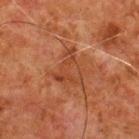Imaged during a routine full-body skin examination; the lesion was not biopsied and no histopathology is available. Approximately 4 mm at its widest. A male subject roughly 80 years of age. From the upper back. Automated tile analysis of the lesion measured a normalized border contrast of about 5.5. The software also gave a border-irregularity index near 7/10 and peripheral color asymmetry of about 1. And it measured an automated nevus-likeness rating near 0 out of 100 and a lesion-detection confidence of about 100/100. Cropped from a total-body skin-imaging series; the visible field is about 15 mm. This is a cross-polarized tile.